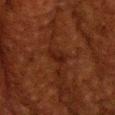biopsy status=no biopsy performed (imaged during a skin exam) | automated lesion analysis=a lesion color around L≈16 a*≈19 b*≈21 in CIELAB, roughly 5 lightness units darker than nearby skin, and a normalized lesion–skin contrast near 6.5; border irregularity of about 3 on a 0–10 scale, internal color variation of about 1 on a 0–10 scale, and radial color variation of about 0.5; a classifier nevus-likeness of about 0/100 and lesion-presence confidence of about 100/100 | subject=male, roughly 60 years of age | tile lighting=cross-polarized illumination | location=the head or neck | lesion diameter=~3 mm (longest diameter) | acquisition=total-body-photography crop, ~15 mm field of view.